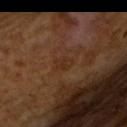Context: A 15 mm close-up tile from a total-body photography series done for melanoma screening. Imaged with cross-polarized lighting. Approximately 2.5 mm at its widest. Automated tile analysis of the lesion measured a shape eccentricity near 0.75 and a shape-asymmetry score of about 0.3 (0 = symmetric). A male patient roughly 65 years of age.